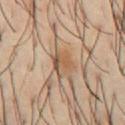No biopsy was performed on this lesion — it was imaged during a full skin examination and was not determined to be concerning.
About 2.5 mm across.
A roughly 15 mm field-of-view crop from a total-body skin photograph.
The total-body-photography lesion software estimated border irregularity of about 2 on a 0–10 scale and radial color variation of about 3.
On the chest.
A male subject, approximately 40 years of age.